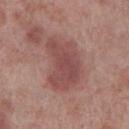No biopsy was performed on this lesion — it was imaged during a full skin examination and was not determined to be concerning. This image is a 15 mm lesion crop taken from a total-body photograph. The lesion's longest dimension is about 6.5 mm. Captured under white-light illumination. Located on the right lower leg. A male patient, aged approximately 70.